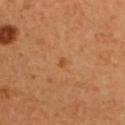lesion size: ≈1 mm
site: the upper back
tile lighting: cross-polarized illumination
image: ~15 mm tile from a whole-body skin photo
subject: female, approximately 40 years of age